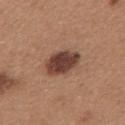| feature | finding |
|---|---|
| follow-up | no biopsy performed (imaged during a skin exam) |
| location | the upper back |
| patient | female, aged around 30 |
| image | total-body-photography crop, ~15 mm field of view |
| diameter | ≈4.5 mm |
| automated metrics | an average lesion color of about L≈41 a*≈21 b*≈25 (CIELAB), about 16 CIELAB-L* units darker than the surrounding skin, and a normalized lesion–skin contrast near 12.5; a border-irregularity rating of about 1.5/10, internal color variation of about 3.5 on a 0–10 scale, and a peripheral color-asymmetry measure near 1; a nevus-likeness score of about 85/100 and lesion-presence confidence of about 100/100 |
| illumination | white-light illumination |